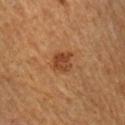A lesion tile, about 15 mm wide, cut from a 3D total-body photograph. The tile uses cross-polarized illumination. A male patient approximately 65 years of age. On the arm. The recorded lesion diameter is about 2.5 mm.